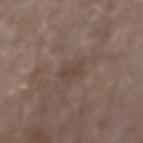Recorded during total-body skin imaging; not selected for excision or biopsy. A male subject aged 53–57. A 15 mm close-up tile from a total-body photography series done for melanoma screening. This is a white-light tile. The lesion-visualizer software estimated an area of roughly 4.5 mm², an outline eccentricity of about 0.45 (0 = round, 1 = elongated), and a shape-asymmetry score of about 0.3 (0 = symmetric). The analysis additionally found roughly 5 lightness units darker than nearby skin and a normalized lesion–skin contrast near 5. It also reported a border-irregularity rating of about 3/10. It also reported a nevus-likeness score of about 0/100 and lesion-presence confidence of about 100/100. Located on the right forearm. Longest diameter approximately 2.5 mm.